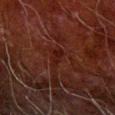Recorded during total-body skin imaging; not selected for excision or biopsy. Captured under cross-polarized illumination. A male subject, about 80 years old. The lesion-visualizer software estimated a mean CIELAB color near L≈14 a*≈21 b*≈18, about 4 CIELAB-L* units darker than the surrounding skin, and a lesion-to-skin contrast of about 6.5 (normalized; higher = more distinct). And it measured a nevus-likeness score of about 0/100. A 15 mm close-up extracted from a 3D total-body photography capture. On the arm. Measured at roughly 3 mm in maximum diameter.